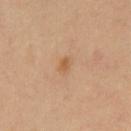Q: What is the imaging modality?
A: total-body-photography crop, ~15 mm field of view
Q: What are the patient's age and sex?
A: male, in their mid-50s
Q: Where on the body is the lesion?
A: the chest